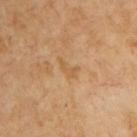Imaged during a routine full-body skin examination; the lesion was not biopsied and no histopathology is available. The lesion-visualizer software estimated an area of roughly 3 mm² and an eccentricity of roughly 0.9. It also reported an average lesion color of about L≈56 a*≈18 b*≈38 (CIELAB), a lesion–skin lightness drop of about 6, and a normalized lesion–skin contrast near 5. The analysis additionally found internal color variation of about 0 on a 0–10 scale and peripheral color asymmetry of about 0. The tile uses cross-polarized illumination. The subject is a male in their mid- to late 60s. Measured at roughly 2.5 mm in maximum diameter. Cropped from a total-body skin-imaging series; the visible field is about 15 mm.